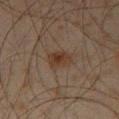No biopsy was performed on this lesion — it was imaged during a full skin examination and was not determined to be concerning. A region of skin cropped from a whole-body photographic capture, roughly 15 mm wide. A male patient, aged around 45. On the left thigh.